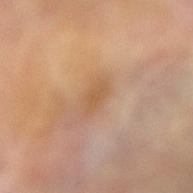Acquisition and patient details:
The lesion is on the right forearm. Imaged with cross-polarized lighting. Measured at roughly 3 mm in maximum diameter. A 15 mm close-up tile from a total-body photography series done for melanoma screening. A female subject in their mid- to late 70s.